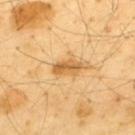Impression:
Imaged during a routine full-body skin examination; the lesion was not biopsied and no histopathology is available.
Acquisition and patient details:
The total-body-photography lesion software estimated a mean CIELAB color near L≈64 a*≈21 b*≈47, a lesion–skin lightness drop of about 12, and a normalized lesion–skin contrast near 8. It also reported a border-irregularity index near 2.5/10, internal color variation of about 4.5 on a 0–10 scale, and a peripheral color-asymmetry measure near 1.5. About 3.5 mm across. This is a cross-polarized tile. Cropped from a total-body skin-imaging series; the visible field is about 15 mm. A male patient in their mid- to late 60s. From the back.